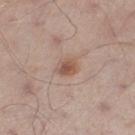Q: Who is the patient?
A: male, about 35 years old
Q: How large is the lesion?
A: ~2.5 mm (longest diameter)
Q: What kind of image is this?
A: 15 mm crop, total-body photography
Q: Illumination type?
A: white-light illumination
Q: Lesion location?
A: the right lower leg
Q: Automated lesion metrics?
A: an area of roughly 4.5 mm², an eccentricity of roughly 0.65, and a shape-asymmetry score of about 0.25 (0 = symmetric); a border-irregularity index near 2/10, a color-variation rating of about 3.5/10, and peripheral color asymmetry of about 1